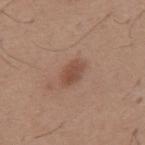Clinical impression:
Recorded during total-body skin imaging; not selected for excision or biopsy.
Context:
The tile uses white-light illumination. Automated tile analysis of the lesion measured an area of roughly 5 mm² and an outline eccentricity of about 0.8 (0 = round, 1 = elongated). Approximately 3 mm at its widest. A roughly 15 mm field-of-view crop from a total-body skin photograph. A male subject, roughly 65 years of age. On the mid back.